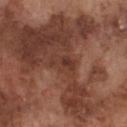image: 15 mm crop, total-body photography
patient: male, in their mid- to late 70s
body site: the chest
tile lighting: white-light